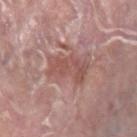Notes:
• follow-up: imaged on a skin check; not biopsied
• lesion diameter: about 5 mm
• illumination: white-light illumination
• imaging modality: ~15 mm crop, total-body skin-cancer survey
• patient: male, aged 38–42
• body site: the right lower leg
• automated metrics: an eccentricity of roughly 0.85 and a symmetry-axis asymmetry near 0.25; an automated nevus-likeness rating near 0 out of 100